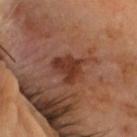anatomic site: the head or neck | acquisition: total-body-photography crop, ~15 mm field of view | lesion diameter: ~4 mm (longest diameter) | illumination: cross-polarized | patient: male, in their 60s | histopathology: an actinic keratosis — a lesion of indeterminate malignant potential.